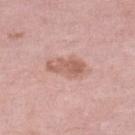lighting: white-light illumination | image source: ~15 mm tile from a whole-body skin photo | subject: female, in their 70s | anatomic site: the left lower leg | automated metrics: an automated nevus-likeness rating near 10 out of 100 and lesion-presence confidence of about 100/100 | size: about 4.5 mm.